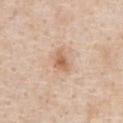Assessment:
Imaged during a routine full-body skin examination; the lesion was not biopsied and no histopathology is available.
Background:
A male subject roughly 60 years of age. The lesion is on the front of the torso. The lesion's longest dimension is about 3 mm. This image is a 15 mm lesion crop taken from a total-body photograph. Captured under white-light illumination. An algorithmic analysis of the crop reported an automated nevus-likeness rating near 35 out of 100.